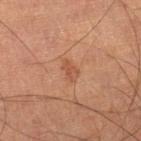Case summary:
* follow-up · no biopsy performed (imaged during a skin exam)
* image-analysis metrics · an area of roughly 3.5 mm², an eccentricity of roughly 0.8, and two-axis asymmetry of about 0.25
* lighting · cross-polarized illumination
* imaging modality · total-body-photography crop, ~15 mm field of view
* subject · male, roughly 60 years of age
* anatomic site · the right lower leg